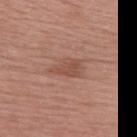The lesion was tiled from a total-body skin photograph and was not biopsied. The patient is a female in their 50s. Cropped from a total-body skin-imaging series; the visible field is about 15 mm. Located on the upper back. About 3.5 mm across. An algorithmic analysis of the crop reported a footprint of about 6 mm², a shape eccentricity near 0.8, and a shape-asymmetry score of about 0.4 (0 = symmetric). The analysis additionally found roughly 8 lightness units darker than nearby skin and a normalized lesion–skin contrast near 6. And it measured a border-irregularity index near 4/10, internal color variation of about 1.5 on a 0–10 scale, and peripheral color asymmetry of about 0.5. It also reported a nevus-likeness score of about 5/100 and lesion-presence confidence of about 100/100. Imaged with white-light lighting.